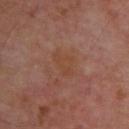Assessment:
The lesion was photographed on a routine skin check and not biopsied; there is no pathology result.
Background:
Cropped from a total-body skin-imaging series; the visible field is about 15 mm. Longest diameter approximately 3.5 mm. The subject is approximately 55 years of age. Located on the upper back.